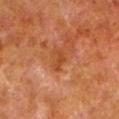follow-up=no biopsy performed (imaged during a skin exam)
body site=the left lower leg
image=~15 mm tile from a whole-body skin photo
patient=male, aged approximately 80